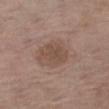<record>
<biopsy_status>not biopsied; imaged during a skin examination</biopsy_status>
<lighting>white-light</lighting>
<lesion_size>
  <long_diameter_mm_approx>4.0</long_diameter_mm_approx>
</lesion_size>
<automated_metrics>
  <border_irregularity_0_10>3.0</border_irregularity_0_10>
  <peripheral_color_asymmetry>0.5</peripheral_color_asymmetry>
  <nevus_likeness_0_100>5</nevus_likeness_0_100>
</automated_metrics>
<image>
  <source>total-body photography crop</source>
  <field_of_view_mm>15</field_of_view_mm>
</image>
<patient>
  <sex>male</sex>
  <age_approx>75</age_approx>
</patient>
<site>right thigh</site>
</record>